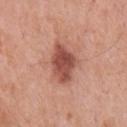Case summary:
• biopsy status — catalogued during a skin exam; not biopsied
• patient — male, approximately 70 years of age
• body site — the chest
• image source — total-body-photography crop, ~15 mm field of view